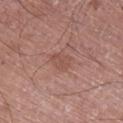The lesion was photographed on a routine skin check and not biopsied; there is no pathology result.
A 15 mm close-up tile from a total-body photography series done for melanoma screening.
Imaged with white-light lighting.
The lesion's longest dimension is about 3 mm.
The subject is a male in their mid-70s.
The lesion is on the right lower leg.